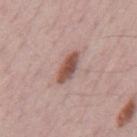This lesion was catalogued during total-body skin photography and was not selected for biopsy.
A 15 mm close-up tile from a total-body photography series done for melanoma screening.
An algorithmic analysis of the crop reported a shape-asymmetry score of about 0.2 (0 = symmetric). The analysis additionally found a mean CIELAB color near L≈52 a*≈21 b*≈25, roughly 14 lightness units darker than nearby skin, and a normalized border contrast of about 9.5. The software also gave a nevus-likeness score of about 95/100 and lesion-presence confidence of about 100/100.
Measured at roughly 4 mm in maximum diameter.
This is a white-light tile.
A male subject, approximately 70 years of age.
From the mid back.